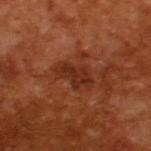The lesion was tiled from a total-body skin photograph and was not biopsied.
This image is a 15 mm lesion crop taken from a total-body photograph.
The recorded lesion diameter is about 4.5 mm.
Imaged with cross-polarized lighting.
The lesion-visualizer software estimated a mean CIELAB color near L≈30 a*≈27 b*≈31 and about 8 CIELAB-L* units darker than the surrounding skin. The analysis additionally found an automated nevus-likeness rating near 20 out of 100 and a lesion-detection confidence of about 100/100.
A male patient about 65 years old.